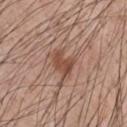Captured during whole-body skin photography for melanoma surveillance; the lesion was not biopsied. A lesion tile, about 15 mm wide, cut from a 3D total-body photograph. Located on the chest. The subject is a male about 55 years old.